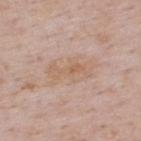Cropped from a total-body skin-imaging series; the visible field is about 15 mm. A male subject aged 48 to 52. Measured at roughly 6 mm in maximum diameter. Located on the back. This is a white-light tile. Automated image analysis of the tile measured an area of roughly 13 mm². The analysis additionally found a nevus-likeness score of about 0/100.